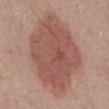Assessment: Recorded during total-body skin imaging; not selected for excision or biopsy. Image and clinical context: The recorded lesion diameter is about 10 mm. Cropped from a whole-body photographic skin survey; the tile spans about 15 mm. This is a white-light tile. The lesion is on the abdomen. The subject is a male approximately 70 years of age.